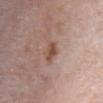– subject: female, about 45 years old
– illumination: white-light illumination
– location: the chest
– diameter: ≈2.5 mm
– imaging modality: 15 mm crop, total-body photography
– automated lesion analysis: border irregularity of about 2 on a 0–10 scale, a within-lesion color-variation index near 3.5/10, and peripheral color asymmetry of about 1